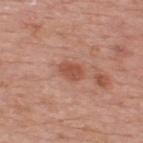No biopsy was performed on this lesion — it was imaged during a full skin examination and was not determined to be concerning.
A 15 mm crop from a total-body photograph taken for skin-cancer surveillance.
The lesion is on the upper back.
An algorithmic analysis of the crop reported an eccentricity of roughly 0.75 and a shape-asymmetry score of about 0.2 (0 = symmetric). The analysis additionally found a border-irregularity index near 1.5/10 and a within-lesion color-variation index near 1.5/10. The analysis additionally found a lesion-detection confidence of about 100/100.
A male patient, aged around 75.
Longest diameter approximately 3 mm.